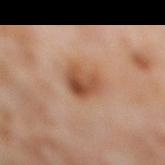A roughly 15 mm field-of-view crop from a total-body skin photograph. A female patient, in their mid-50s. On the leg.